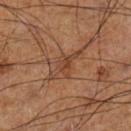{"biopsy_status": "not biopsied; imaged during a skin examination", "lesion_size": {"long_diameter_mm_approx": 5.0}, "patient": {"sex": "male", "age_approx": 60}, "site": "left lower leg", "image": {"source": "total-body photography crop", "field_of_view_mm": 15}}